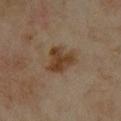Captured during whole-body skin photography for melanoma surveillance; the lesion was not biopsied.
The lesion is on the left forearm.
This is a cross-polarized tile.
A female subject roughly 35 years of age.
An algorithmic analysis of the crop reported a lesion color around L≈38 a*≈15 b*≈29 in CIELAB. The software also gave a border-irregularity index near 3/10, a color-variation rating of about 3.5/10, and peripheral color asymmetry of about 1. The software also gave a lesion-detection confidence of about 100/100.
A close-up tile cropped from a whole-body skin photograph, about 15 mm across.
The recorded lesion diameter is about 4 mm.